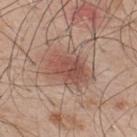Part of a total-body skin-imaging series; this lesion was reviewed on a skin check and was not flagged for biopsy.
Located on the upper back.
The subject is a male aged 48 to 52.
A roughly 15 mm field-of-view crop from a total-body skin photograph.